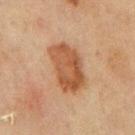Part of a total-body skin-imaging series; this lesion was reviewed on a skin check and was not flagged for biopsy. The lesion is located on the mid back. This image is a 15 mm lesion crop taken from a total-body photograph. The tile uses cross-polarized illumination. A male subject aged approximately 70.